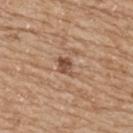| field | value |
|---|---|
| follow-up | no biopsy performed (imaged during a skin exam) |
| diameter | ≈3 mm |
| image source | ~15 mm crop, total-body skin-cancer survey |
| site | the back |
| lighting | white-light |
| patient | female, roughly 70 years of age |
| TBP lesion metrics | internal color variation of about 4 on a 0–10 scale and peripheral color asymmetry of about 1.5; a nevus-likeness score of about 70/100 and a detector confidence of about 100 out of 100 that the crop contains a lesion |